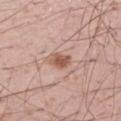The lesion is on the left thigh.
The lesion's longest dimension is about 2.5 mm.
A roughly 15 mm field-of-view crop from a total-body skin photograph.
The subject is a male aged around 55.